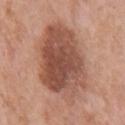imaging modality = ~15 mm crop, total-body skin-cancer survey
body site = the chest
illumination = white-light illumination
diameter = ~9.5 mm (longest diameter)
subject = male, aged around 70